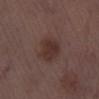Assessment:
The lesion was tiled from a total-body skin photograph and was not biopsied.
Image and clinical context:
A 15 mm close-up extracted from a 3D total-body photography capture. On the left lower leg. About 4 mm across. The subject is a male in their 70s.